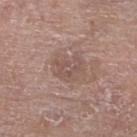| feature | finding |
|---|---|
| notes | no biopsy performed (imaged during a skin exam) |
| imaging modality | ~15 mm tile from a whole-body skin photo |
| tile lighting | white-light illumination |
| site | the right lower leg |
| subject | male, in their 80s |